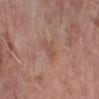The patient is a female aged around 65.
Longest diameter approximately 3 mm.
The tile uses white-light illumination.
From the right forearm.
A region of skin cropped from a whole-body photographic capture, roughly 15 mm wide.